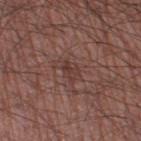The lesion was tiled from a total-body skin photograph and was not biopsied.
A close-up tile cropped from a whole-body skin photograph, about 15 mm across.
The lesion's longest dimension is about 2.5 mm.
From the right thigh.
The patient is a male approximately 60 years of age.
Captured under white-light illumination.